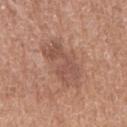<tbp_lesion>
<biopsy_status>not biopsied; imaged during a skin examination</biopsy_status>
<image>
  <source>total-body photography crop</source>
  <field_of_view_mm>15</field_of_view_mm>
</image>
<patient>
  <sex>female</sex>
  <age_approx>55</age_approx>
</patient>
<site>arm</site>
</tbp_lesion>